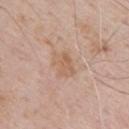biopsy status: total-body-photography surveillance lesion; no biopsy | image: ~15 mm crop, total-body skin-cancer survey | size: ~3.5 mm (longest diameter) | site: the upper back | lighting: white-light | subject: male, in their 70s | image-analysis metrics: an area of roughly 6.5 mm² and a symmetry-axis asymmetry near 0.35; a border-irregularity index near 4/10; an automated nevus-likeness rating near 0 out of 100 and a lesion-detection confidence of about 100/100.